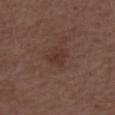This lesion was catalogued during total-body skin photography and was not selected for biopsy.
Automated image analysis of the tile measured a lesion color around L≈34 a*≈19 b*≈23 in CIELAB, a lesion–skin lightness drop of about 6, and a lesion-to-skin contrast of about 5.5 (normalized; higher = more distinct). It also reported border irregularity of about 3 on a 0–10 scale and a peripheral color-asymmetry measure near 1. And it measured a nevus-likeness score of about 10/100 and a lesion-detection confidence of about 100/100.
A 15 mm close-up extracted from a 3D total-body photography capture.
Measured at roughly 2.5 mm in maximum diameter.
The lesion is located on the right upper arm.
Captured under white-light illumination.
A male subject, aged 68 to 72.